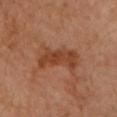<record>
<biopsy_status>not biopsied; imaged during a skin examination</biopsy_status>
<lesion_size>
  <long_diameter_mm_approx>6.0</long_diameter_mm_approx>
</lesion_size>
<patient>
  <sex>female</sex>
  <age_approx>65</age_approx>
</patient>
<image>
  <source>total-body photography crop</source>
  <field_of_view_mm>15</field_of_view_mm>
</image>
<lighting>cross-polarized</lighting>
<site>left forearm</site>
</record>